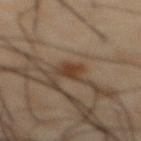{"biopsy_status": "not biopsied; imaged during a skin examination", "image": {"source": "total-body photography crop", "field_of_view_mm": 15}, "site": "abdomen", "lesion_size": {"long_diameter_mm_approx": 2.5}, "automated_metrics": {"area_mm2_approx": 4.0, "eccentricity": 0.65, "shape_asymmetry": 0.35, "border_irregularity_0_10": 3.0, "peripheral_color_asymmetry": 0.5, "nevus_likeness_0_100": 85, "lesion_detection_confidence_0_100": 100}, "patient": {"sex": "male", "age_approx": 50}}